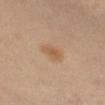The lesion was photographed on a routine skin check and not biopsied; there is no pathology result. Cropped from a total-body skin-imaging series; the visible field is about 15 mm. The patient is a female about 40 years old. The tile uses cross-polarized illumination. The recorded lesion diameter is about 3 mm. The lesion is on the right leg.